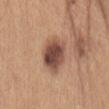| feature | finding |
|---|---|
| biopsy status | imaged on a skin check; not biopsied |
| lesion size | ≈5 mm |
| body site | the abdomen |
| illumination | white-light |
| automated metrics | an average lesion color of about L≈48 a*≈20 b*≈26 (CIELAB), about 16 CIELAB-L* units darker than the surrounding skin, and a normalized lesion–skin contrast near 11.5 |
| acquisition | 15 mm crop, total-body photography |
| subject | female, aged approximately 35 |